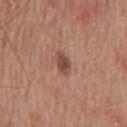Part of a total-body skin-imaging series; this lesion was reviewed on a skin check and was not flagged for biopsy. On the front of the torso. The lesion's longest dimension is about 2.5 mm. Captured under white-light illumination. A male patient, in their mid- to late 60s. Automated image analysis of the tile measured a mean CIELAB color near L≈47 a*≈23 b*≈27, a lesion–skin lightness drop of about 11, and a normalized border contrast of about 8. And it measured a nevus-likeness score of about 85/100 and a detector confidence of about 100 out of 100 that the crop contains a lesion. A close-up tile cropped from a whole-body skin photograph, about 15 mm across.